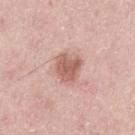The lesion's longest dimension is about 3.5 mm. Captured under white-light illumination. A male subject, in their mid- to late 40s. On the left upper arm. The total-body-photography lesion software estimated a mean CIELAB color near L≈59 a*≈23 b*≈27, about 12 CIELAB-L* units darker than the surrounding skin, and a normalized border contrast of about 8. It also reported a border-irregularity index near 2.5/10, a within-lesion color-variation index near 3/10, and radial color variation of about 1. The software also gave an automated nevus-likeness rating near 40 out of 100 and a lesion-detection confidence of about 100/100. Cropped from a whole-body photographic skin survey; the tile spans about 15 mm.